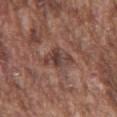Clinical impression: Part of a total-body skin-imaging series; this lesion was reviewed on a skin check and was not flagged for biopsy. Background: A close-up tile cropped from a whole-body skin photograph, about 15 mm across. The subject is a male aged 73 to 77. From the chest.